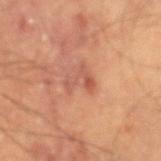Q: Was a biopsy performed?
A: catalogued during a skin exam; not biopsied
Q: Illumination type?
A: cross-polarized illumination
Q: Who is the patient?
A: male, roughly 60 years of age
Q: How large is the lesion?
A: about 3 mm
Q: Lesion location?
A: the left forearm
Q: How was this image acquired?
A: total-body-photography crop, ~15 mm field of view